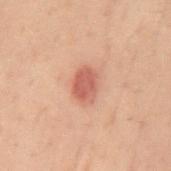Clinical impression:
No biopsy was performed on this lesion — it was imaged during a full skin examination and was not determined to be concerning.
Acquisition and patient details:
A male patient, aged 38–42. Imaged with cross-polarized lighting. Approximately 3.5 mm at its widest. The lesion is on the mid back. Automated tile analysis of the lesion measured border irregularity of about 1.5 on a 0–10 scale and radial color variation of about 0.5. And it measured a classifier nevus-likeness of about 95/100 and a detector confidence of about 100 out of 100 that the crop contains a lesion. A roughly 15 mm field-of-view crop from a total-body skin photograph.